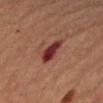follow-up = no biopsy performed (imaged during a skin exam)
image source = total-body-photography crop, ~15 mm field of view
diameter = ~3.5 mm (longest diameter)
location = the right forearm
patient = female, aged approximately 70
illumination = cross-polarized